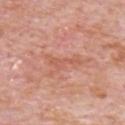notes = total-body-photography surveillance lesion; no biopsy.